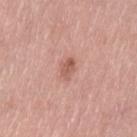notes=imaged on a skin check; not biopsied
image source=total-body-photography crop, ~15 mm field of view
illumination=white-light illumination
subject=female, aged 38–42
size=≈2.5 mm
anatomic site=the left thigh